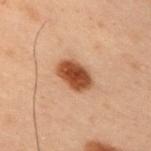| key | value |
|---|---|
| follow-up | catalogued during a skin exam; not biopsied |